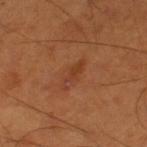biopsy status — catalogued during a skin exam; not biopsied
tile lighting — cross-polarized illumination
diameter — ~4 mm (longest diameter)
patient — male, approximately 55 years of age
TBP lesion metrics — roughly 6 lightness units darker than nearby skin and a normalized border contrast of about 5.5; an automated nevus-likeness rating near 0 out of 100
imaging modality — ~15 mm crop, total-body skin-cancer survey
body site — the left thigh